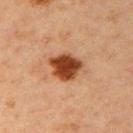Assessment:
Recorded during total-body skin imaging; not selected for excision or biopsy.
Clinical summary:
A female patient in their mid- to late 40s. The lesion is on the right upper arm. Imaged with cross-polarized lighting. A region of skin cropped from a whole-body photographic capture, roughly 15 mm wide.